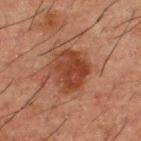notes = total-body-photography surveillance lesion; no biopsy | lesion diameter = ~5 mm (longest diameter) | automated lesion analysis = a lesion area of about 15 mm², an eccentricity of roughly 0.6, and a symmetry-axis asymmetry near 0.25; a border-irregularity index near 2.5/10 and peripheral color asymmetry of about 1 | site = the upper back | imaging modality = ~15 mm crop, total-body skin-cancer survey | subject = male, aged 48–52 | tile lighting = cross-polarized.